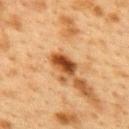Imaged during a routine full-body skin examination; the lesion was not biopsied and no histopathology is available. Longest diameter approximately 3.5 mm. The lesion-visualizer software estimated a mean CIELAB color near L≈40 a*≈23 b*≈36 and a normalized lesion–skin contrast near 12.5. From the upper back. A lesion tile, about 15 mm wide, cut from a 3D total-body photograph. The patient is a female aged around 40.